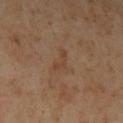The lesion was photographed on a routine skin check and not biopsied; there is no pathology result. A female subject aged 48–52. An algorithmic analysis of the crop reported a mean CIELAB color near L≈43 a*≈18 b*≈31. It also reported border irregularity of about 6.5 on a 0–10 scale, a color-variation rating of about 0/10, and radial color variation of about 0. And it measured a nevus-likeness score of about 0/100 and lesion-presence confidence of about 100/100. Captured under cross-polarized illumination. A close-up tile cropped from a whole-body skin photograph, about 15 mm across. On the left lower leg.